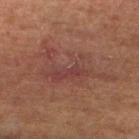Part of a total-body skin-imaging series; this lesion was reviewed on a skin check and was not flagged for biopsy. The lesion is located on the left lower leg. Automated image analysis of the tile measured a classifier nevus-likeness of about 0/100 and a detector confidence of about 95 out of 100 that the crop contains a lesion. A close-up tile cropped from a whole-body skin photograph, about 15 mm across. A male subject, in their mid-60s. Imaged with cross-polarized lighting. The lesion's longest dimension is about 9 mm.